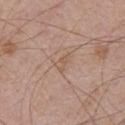Captured during whole-body skin photography for melanoma surveillance; the lesion was not biopsied. A male patient, approximately 70 years of age. A 15 mm crop from a total-body photograph taken for skin-cancer surveillance. From the front of the torso.